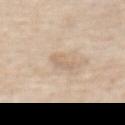biopsy status: no biopsy performed (imaged during a skin exam); tile lighting: white-light illumination; subject: male, about 70 years old; TBP lesion metrics: a lesion area of about 4.5 mm² and an outline eccentricity of about 0.9 (0 = round, 1 = elongated); image source: total-body-photography crop, ~15 mm field of view; anatomic site: the abdomen.